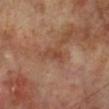Q: Was this lesion biopsied?
A: imaged on a skin check; not biopsied
Q: What is the imaging modality?
A: 15 mm crop, total-body photography
Q: What are the patient's age and sex?
A: male, aged approximately 70
Q: Lesion location?
A: the left lower leg
Q: Automated lesion metrics?
A: a footprint of about 3 mm² and an outline eccentricity of about 0.9 (0 = round, 1 = elongated); a lesion color around L≈43 a*≈22 b*≈30 in CIELAB, roughly 6 lightness units darker than nearby skin, and a normalized border contrast of about 5.5; a border-irregularity rating of about 3.5/10 and a color-variation rating of about 0.5/10
Q: How large is the lesion?
A: ≈3 mm
Q: What lighting was used for the tile?
A: cross-polarized